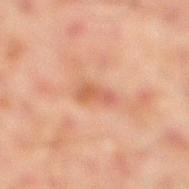Case summary:
- workup · total-body-photography surveillance lesion; no biopsy
- lesion diameter · about 3.5 mm
- body site · the right lower leg
- TBP lesion metrics · a shape eccentricity near 0.9 and a shape-asymmetry score of about 0.35 (0 = symmetric)
- illumination · cross-polarized illumination
- acquisition · total-body-photography crop, ~15 mm field of view
- patient · male, about 45 years old